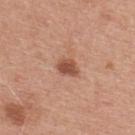workup = imaged on a skin check; not biopsied | imaging modality = ~15 mm tile from a whole-body skin photo | location = the upper back | diameter = about 2.5 mm | tile lighting = white-light | patient = male, aged around 30.